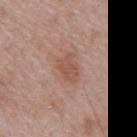No biopsy was performed on this lesion — it was imaged during a full skin examination and was not determined to be concerning. This image is a 15 mm lesion crop taken from a total-body photograph. Captured under white-light illumination. About 3.5 mm across. The subject is a male in their mid- to late 60s. Located on the chest.